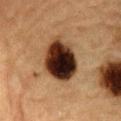No biopsy was performed on this lesion — it was imaged during a full skin examination and was not determined to be concerning. The lesion is on the abdomen. A 15 mm crop from a total-body photograph taken for skin-cancer surveillance. A male patient, aged approximately 85 or older. The recorded lesion diameter is about 6 mm. The lesion-visualizer software estimated a lesion area of about 19 mm², an eccentricity of roughly 0.7, and a shape-asymmetry score of about 0.1 (0 = symmetric). The software also gave a lesion color around L≈25 a*≈17 b*≈24 in CIELAB and a lesion–skin lightness drop of about 25. Captured under cross-polarized illumination.